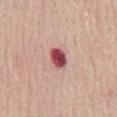subject: female, about 65 years old
image: 15 mm crop, total-body photography
tile lighting: white-light
anatomic site: the mid back
automated lesion analysis: an area of roughly 5 mm² and a symmetry-axis asymmetry near 0.2; a border-irregularity index near 1.5/10, internal color variation of about 5 on a 0–10 scale, and a peripheral color-asymmetry measure near 1.5